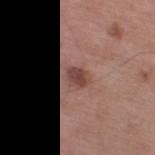No biopsy was performed on this lesion — it was imaged during a full skin examination and was not determined to be concerning.
The lesion's longest dimension is about 2.5 mm.
The lesion-visualizer software estimated border irregularity of about 1.5 on a 0–10 scale, internal color variation of about 2 on a 0–10 scale, and peripheral color asymmetry of about 0.5.
This is a white-light tile.
On the leg.
A 15 mm crop from a total-body photograph taken for skin-cancer surveillance.
A male subject aged around 70.